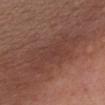<lesion>
  <biopsy_status>not biopsied; imaged during a skin examination</biopsy_status>
</lesion>